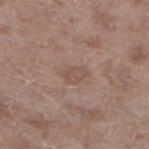Q: Is there a histopathology result?
A: catalogued during a skin exam; not biopsied
Q: Who is the patient?
A: male, aged 43 to 47
Q: What is the imaging modality?
A: ~15 mm tile from a whole-body skin photo
Q: How large is the lesion?
A: ≈3.5 mm
Q: Where on the body is the lesion?
A: the left lower leg
Q: What lighting was used for the tile?
A: white-light illumination